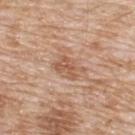Impression: No biopsy was performed on this lesion — it was imaged during a full skin examination and was not determined to be concerning. Acquisition and patient details: The lesion is on the upper back. A 15 mm crop from a total-body photograph taken for skin-cancer surveillance. A male subject aged approximately 80.